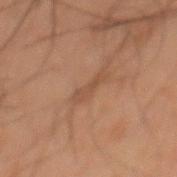Case summary:
• workup · total-body-photography surveillance lesion; no biopsy
• acquisition · total-body-photography crop, ~15 mm field of view
• image-analysis metrics · a lesion area of about 3 mm², an eccentricity of roughly 0.95, and a shape-asymmetry score of about 0.35 (0 = symmetric); an average lesion color of about L≈37 a*≈16 b*≈25 (CIELAB), roughly 5 lightness units darker than nearby skin, and a normalized lesion–skin contrast near 5; border irregularity of about 4.5 on a 0–10 scale and a within-lesion color-variation index near 0/10; an automated nevus-likeness rating near 0 out of 100 and lesion-presence confidence of about 55/100
• illumination · cross-polarized
• subject · male, in their 60s
• location · the back
• lesion size · ~3.5 mm (longest diameter)